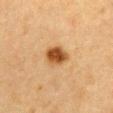Assessment: The lesion was photographed on a routine skin check and not biopsied; there is no pathology result. Clinical summary: A female patient roughly 55 years of age. A 15 mm crop from a total-body photograph taken for skin-cancer surveillance. The lesion is located on the chest. Imaged with cross-polarized lighting.